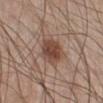Captured during whole-body skin photography for melanoma surveillance; the lesion was not biopsied.
The total-body-photography lesion software estimated a lesion area of about 9 mm² and an outline eccentricity of about 0.6 (0 = round, 1 = elongated). And it measured border irregularity of about 2 on a 0–10 scale and a within-lesion color-variation index near 3.5/10. The software also gave a classifier nevus-likeness of about 90/100 and a lesion-detection confidence of about 100/100.
This is a white-light tile.
About 3.5 mm across.
The lesion is located on the right lower leg.
Cropped from a whole-body photographic skin survey; the tile spans about 15 mm.
A male patient aged 58 to 62.